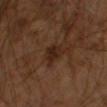Clinical impression:
This lesion was catalogued during total-body skin photography and was not selected for biopsy.
Background:
A 15 mm close-up tile from a total-body photography series done for melanoma screening. A male patient approximately 60 years of age. The tile uses cross-polarized illumination. From the left arm. An algorithmic analysis of the crop reported an average lesion color of about L≈23 a*≈16 b*≈24 (CIELAB). And it measured an automated nevus-likeness rating near 0 out of 100.